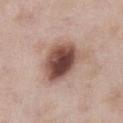Q: Is there a histopathology result?
A: no biopsy performed (imaged during a skin exam)
Q: How was this image acquired?
A: 15 mm crop, total-body photography
Q: Patient demographics?
A: male, aged 53–57
Q: Where on the body is the lesion?
A: the abdomen
Q: How large is the lesion?
A: ~5.5 mm (longest diameter)
Q: What lighting was used for the tile?
A: white-light
Q: Automated lesion metrics?
A: a lesion–skin lightness drop of about 18 and a normalized border contrast of about 12; a classifier nevus-likeness of about 90/100